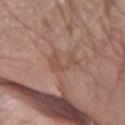Recorded during total-body skin imaging; not selected for excision or biopsy. The tile uses white-light illumination. Automated tile analysis of the lesion measured a mean CIELAB color near L≈50 a*≈20 b*≈26, roughly 6 lightness units darker than nearby skin, and a normalized lesion–skin contrast near 5. The analysis additionally found a border-irregularity rating of about 7.5/10, a color-variation rating of about 1.5/10, and radial color variation of about 0.5. And it measured a classifier nevus-likeness of about 0/100 and lesion-presence confidence of about 95/100. A female subject aged around 75. About 3.5 mm across. A 15 mm close-up tile from a total-body photography series done for melanoma screening. Located on the left forearm.